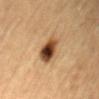  biopsy_status: not biopsied; imaged during a skin examination
  image:
    source: total-body photography crop
    field_of_view_mm: 15
  patient:
    sex: male
    age_approx: 85+
  site: back